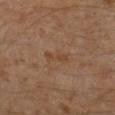| key | value |
|---|---|
| follow-up | imaged on a skin check; not biopsied |
| size | ≈3 mm |
| image | 15 mm crop, total-body photography |
| site | the left leg |
| patient | male, about 30 years old |
| image-analysis metrics | border irregularity of about 3.5 on a 0–10 scale and a color-variation rating of about 0/10; a nevus-likeness score of about 0/100 and a lesion-detection confidence of about 100/100 |
| tile lighting | cross-polarized illumination |